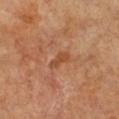{
  "biopsy_status": "not biopsied; imaged during a skin examination",
  "patient": {
    "sex": "female",
    "age_approx": 55
  },
  "lesion_size": {
    "long_diameter_mm_approx": 3.0
  },
  "site": "leg",
  "image": {
    "source": "total-body photography crop",
    "field_of_view_mm": 15
  },
  "lighting": "cross-polarized"
}